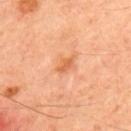workup = no biopsy performed (imaged during a skin exam) | site = the upper back | TBP lesion metrics = a mean CIELAB color near L≈63 a*≈29 b*≈41, a lesion–skin lightness drop of about 9, and a normalized lesion–skin contrast near 6.5; a border-irregularity index near 3/10 and radial color variation of about 0.5 | tile lighting = cross-polarized illumination | lesion diameter = ~2.5 mm (longest diameter) | subject = male, in their 50s | image source = total-body-photography crop, ~15 mm field of view.